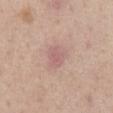biopsy status — imaged on a skin check; not biopsied | TBP lesion metrics — an average lesion color of about L≈60 a*≈21 b*≈22 (CIELAB), a lesion–skin lightness drop of about 7, and a normalized border contrast of about 5; a border-irregularity rating of about 2.5/10, internal color variation of about 2.5 on a 0–10 scale, and radial color variation of about 1; a nevus-likeness score of about 0/100 and a detector confidence of about 100 out of 100 that the crop contains a lesion | location — the mid back | lesion diameter — ≈3 mm | imaging modality — total-body-photography crop, ~15 mm field of view | subject — male, in their mid-50s.